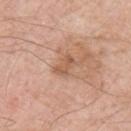Q: Was a biopsy performed?
A: total-body-photography surveillance lesion; no biopsy
Q: What did automated image analysis measure?
A: an average lesion color of about L≈56 a*≈21 b*≈32 (CIELAB) and a lesion-to-skin contrast of about 6 (normalized; higher = more distinct); an automated nevus-likeness rating near 0 out of 100 and lesion-presence confidence of about 100/100
Q: How was the tile lit?
A: white-light illumination
Q: What are the patient's age and sex?
A: male, aged around 60
Q: What is the imaging modality?
A: total-body-photography crop, ~15 mm field of view
Q: What is the anatomic site?
A: the right upper arm